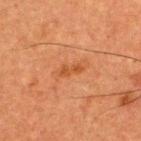No biopsy was performed on this lesion — it was imaged during a full skin examination and was not determined to be concerning.
Captured under cross-polarized illumination.
A male subject aged approximately 50.
A roughly 15 mm field-of-view crop from a total-body skin photograph.
The lesion is on the upper back.